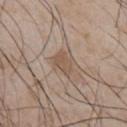| feature | finding |
|---|---|
| lighting | white-light |
| anatomic site | the front of the torso |
| automated metrics | an average lesion color of about L≈53 a*≈15 b*≈27 (CIELAB), about 8 CIELAB-L* units darker than the surrounding skin, and a normalized lesion–skin contrast near 6 |
| patient | male, aged approximately 50 |
| lesion diameter | about 3 mm |
| imaging modality | ~15 mm tile from a whole-body skin photo |